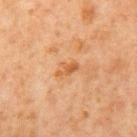| field | value |
|---|---|
| automated lesion analysis | a footprint of about 3 mm² and a symmetry-axis asymmetry near 0.4 |
| lesion size | ≈2.5 mm |
| anatomic site | the mid back |
| lighting | cross-polarized |
| subject | male, aged around 65 |
| imaging modality | ~15 mm crop, total-body skin-cancer survey |